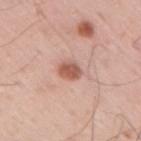follow-up=catalogued during a skin exam; not biopsied | imaging modality=total-body-photography crop, ~15 mm field of view | lesion diameter=about 2.5 mm | image-analysis metrics=a border-irregularity rating of about 2/10, a color-variation rating of about 2.5/10, and radial color variation of about 1 | subject=male, about 35 years old | anatomic site=the right upper arm.